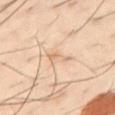– follow-up — total-body-photography surveillance lesion; no biopsy
– patient — male, approximately 40 years of age
– body site — the front of the torso
– acquisition — ~15 mm tile from a whole-body skin photo